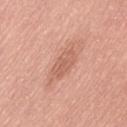Captured during whole-body skin photography for melanoma surveillance; the lesion was not biopsied. Located on the leg. About 3.5 mm across. A male subject, in their 50s. Captured under white-light illumination. Cropped from a whole-body photographic skin survey; the tile spans about 15 mm.